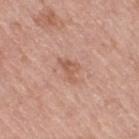No biopsy was performed on this lesion — it was imaged during a full skin examination and was not determined to be concerning.